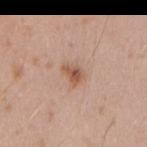{"biopsy_status": "not biopsied; imaged during a skin examination", "lesion_size": {"long_diameter_mm_approx": 2.5}, "automated_metrics": {"cielab_L": 55, "cielab_a": 21, "cielab_b": 30, "vs_skin_darker_L": 11.0, "vs_skin_contrast_norm": 8.0, "nevus_likeness_0_100": 80, "lesion_detection_confidence_0_100": 100}, "patient": {"sex": "male", "age_approx": 35}, "site": "right upper arm", "image": {"source": "total-body photography crop", "field_of_view_mm": 15}}